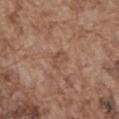Clinical impression:
No biopsy was performed on this lesion — it was imaged during a full skin examination and was not determined to be concerning.
Context:
A male patient aged 73 to 77. The recorded lesion diameter is about 2.5 mm. A lesion tile, about 15 mm wide, cut from a 3D total-body photograph. The lesion-visualizer software estimated an automated nevus-likeness rating near 0 out of 100. From the abdomen.